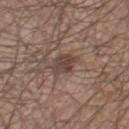Clinical impression: The lesion was tiled from a total-body skin photograph and was not biopsied. Clinical summary: This image is a 15 mm lesion crop taken from a total-body photograph. The lesion-visualizer software estimated a lesion color around L≈43 a*≈15 b*≈22 in CIELAB and a lesion–skin lightness drop of about 10. The software also gave border irregularity of about 3 on a 0–10 scale, a color-variation rating of about 3/10, and a peripheral color-asymmetry measure near 1. A male patient, about 65 years old. About 4 mm across. From the chest. Imaged with white-light lighting.